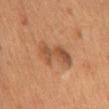| key | value |
|---|---|
| biopsy status | catalogued during a skin exam; not biopsied |
| lesion size | ~5.5 mm (longest diameter) |
| anatomic site | the mid back |
| subject | male, aged around 55 |
| image | ~15 mm tile from a whole-body skin photo |
| lighting | cross-polarized |